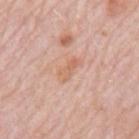Clinical impression: The lesion was tiled from a total-body skin photograph and was not biopsied. Context: The recorded lesion diameter is about 3 mm. The total-body-photography lesion software estimated a within-lesion color-variation index near 0/10 and peripheral color asymmetry of about 0. It also reported a nevus-likeness score of about 0/100. A male subject, about 80 years old. A 15 mm close-up tile from a total-body photography series done for melanoma screening. On the back.